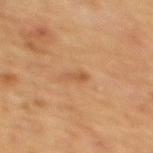* workup: no biopsy performed (imaged during a skin exam)
* tile lighting: cross-polarized illumination
* diameter: ~3 mm (longest diameter)
* automated lesion analysis: a footprint of about 2.5 mm², a shape eccentricity near 0.9, and a symmetry-axis asymmetry near 0.35; a border-irregularity index near 4/10, a color-variation rating of about 0/10, and peripheral color asymmetry of about 0
* imaging modality: 15 mm crop, total-body photography
* body site: the back
* subject: female, roughly 70 years of age